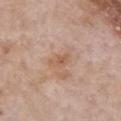follow-up — total-body-photography surveillance lesion; no biopsy
subject — female, roughly 85 years of age or older
tile lighting — white-light illumination
body site — the chest
lesion size — ≈3 mm
automated metrics — a border-irregularity index near 3/10, a color-variation rating of about 3/10, and a peripheral color-asymmetry measure near 1; a nevus-likeness score of about 0/100
acquisition — ~15 mm tile from a whole-body skin photo